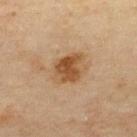Imaged during a routine full-body skin examination; the lesion was not biopsied and no histopathology is available. Located on the upper back. A 15 mm close-up extracted from a 3D total-body photography capture. A male patient aged 43 to 47. About 3.5 mm across.